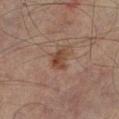Impression: The lesion was tiled from a total-body skin photograph and was not biopsied. Clinical summary: Cropped from a whole-body photographic skin survey; the tile spans about 15 mm. The lesion is on the leg. Longest diameter approximately 3 mm. The patient is a male aged 73 to 77. This is a cross-polarized tile.